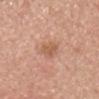Imaged during a routine full-body skin examination; the lesion was not biopsied and no histopathology is available. The subject is a male aged around 60. Imaged with white-light lighting. On the head or neck. Cropped from a total-body skin-imaging series; the visible field is about 15 mm. The lesion-visualizer software estimated a lesion color around L≈58 a*≈22 b*≈32 in CIELAB. The software also gave border irregularity of about 2 on a 0–10 scale, a within-lesion color-variation index near 2.5/10, and peripheral color asymmetry of about 1. Measured at roughly 2.5 mm in maximum diameter.